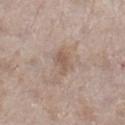Impression: Captured during whole-body skin photography for melanoma surveillance; the lesion was not biopsied. Background: A 15 mm close-up extracted from a 3D total-body photography capture. The patient is a female aged approximately 75. Imaged with white-light lighting. On the right lower leg.